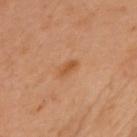Imaged during a routine full-body skin examination; the lesion was not biopsied and no histopathology is available. The patient is a female in their 60s. The tile uses cross-polarized illumination. A roughly 15 mm field-of-view crop from a total-body skin photograph. Automated image analysis of the tile measured a footprint of about 3 mm², a shape eccentricity near 0.85, and a shape-asymmetry score of about 0.3 (0 = symmetric). The analysis additionally found an average lesion color of about L≈46 a*≈21 b*≈35 (CIELAB) and roughly 7 lightness units darker than nearby skin. And it measured a border-irregularity index near 2.5/10. The software also gave a nevus-likeness score of about 45/100 and lesion-presence confidence of about 100/100. On the front of the torso.